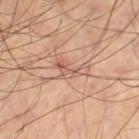{"biopsy_status": "not biopsied; imaged during a skin examination", "lesion_size": {"long_diameter_mm_approx": 4.0}, "patient": {"sex": "male", "age_approx": 60}, "lighting": "cross-polarized", "site": "left thigh", "automated_metrics": {"border_irregularity_0_10": 6.5, "color_variation_0_10": 5.0, "peripheral_color_asymmetry": 1.0}, "image": {"source": "total-body photography crop", "field_of_view_mm": 15}}